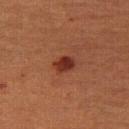lesion_size:
  long_diameter_mm_approx: 2.5
lighting: cross-polarized
image:
  source: total-body photography crop
  field_of_view_mm: 15
site: right thigh
patient:
  sex: female
  age_approx: 55
automated_metrics:
  eccentricity: 0.65
  shape_asymmetry: 0.25
  cielab_L: 28
  cielab_a: 25
  cielab_b: 26
  vs_skin_contrast_norm: 10.0
  lesion_detection_confidence_0_100: 100